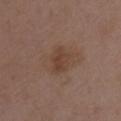biopsy status = catalogued during a skin exam; not biopsied | subject = female, aged approximately 30 | acquisition = ~15 mm crop, total-body skin-cancer survey | site = the left upper arm.